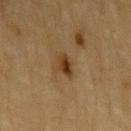Impression:
This lesion was catalogued during total-body skin photography and was not selected for biopsy.
Image and clinical context:
The lesion is on the left upper arm. About 2.5 mm across. A 15 mm close-up extracted from a 3D total-body photography capture. The patient is a male aged 83 or older. Imaged with cross-polarized lighting. The total-body-photography lesion software estimated a lesion color around L≈32 a*≈15 b*≈30 in CIELAB and a lesion-to-skin contrast of about 9 (normalized; higher = more distinct). And it measured a nevus-likeness score of about 95/100 and a detector confidence of about 100 out of 100 that the crop contains a lesion.